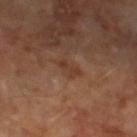A close-up tile cropped from a whole-body skin photograph, about 15 mm across. Captured under cross-polarized illumination. Located on the left lower leg. A male patient, aged 68 to 72.